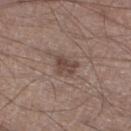Clinical impression:
Captured during whole-body skin photography for melanoma surveillance; the lesion was not biopsied.
Context:
Longest diameter approximately 2.5 mm. A male patient, in their mid-50s. A 15 mm close-up tile from a total-body photography series done for melanoma screening. The lesion is located on the leg.